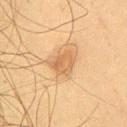Acquisition and patient details: A male patient, in their mid- to late 40s. From the chest. A close-up tile cropped from a whole-body skin photograph, about 15 mm across. Captured under cross-polarized illumination. Measured at roughly 3 mm in maximum diameter.